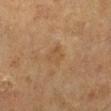No biopsy was performed on this lesion — it was imaged during a full skin examination and was not determined to be concerning.
Imaged with cross-polarized lighting.
Automated tile analysis of the lesion measured a shape eccentricity near 0.45. It also reported border irregularity of about 3 on a 0–10 scale, a color-variation rating of about 2/10, and radial color variation of about 1.
Located on the leg.
Longest diameter approximately 2.5 mm.
This image is a 15 mm lesion crop taken from a total-body photograph.
A male subject, in their mid- to late 80s or older.